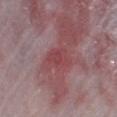This lesion was catalogued during total-body skin photography and was not selected for biopsy.
Cropped from a total-body skin-imaging series; the visible field is about 15 mm.
Located on the right lower leg.
A male patient approximately 65 years of age.
This is a white-light tile.
Approximately 3 mm at its widest.
The total-body-photography lesion software estimated an automated nevus-likeness rating near 0 out of 100 and lesion-presence confidence of about 80/100.